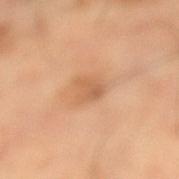No biopsy was performed on this lesion — it was imaged during a full skin examination and was not determined to be concerning.
Captured under cross-polarized illumination.
A region of skin cropped from a whole-body photographic capture, roughly 15 mm wide.
A female patient, aged around 60.
Measured at roughly 3 mm in maximum diameter.
The lesion is on the leg.
An algorithmic analysis of the crop reported a nevus-likeness score of about 0/100.